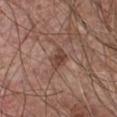From the chest. Imaged with white-light lighting. Longest diameter approximately 3 mm. A male subject aged around 65. A 15 mm close-up extracted from a 3D total-body photography capture.